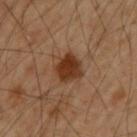Imaged during a routine full-body skin examination; the lesion was not biopsied and no histopathology is available.
The total-body-photography lesion software estimated an automated nevus-likeness rating near 100 out of 100 and a lesion-detection confidence of about 100/100.
The lesion is on the upper back.
The patient is a male in their mid-50s.
A roughly 15 mm field-of-view crop from a total-body skin photograph.
The tile uses cross-polarized illumination.